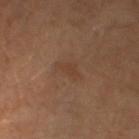Part of a total-body skin-imaging series; this lesion was reviewed on a skin check and was not flagged for biopsy.
A male patient, aged around 30.
Automated image analysis of the tile measured a mean CIELAB color near L≈38 a*≈18 b*≈28, a lesion–skin lightness drop of about 5, and a normalized border contrast of about 5. It also reported a border-irregularity index near 4.5/10, a within-lesion color-variation index near 1/10, and radial color variation of about 0.5.
Located on the left lower leg.
Measured at roughly 3 mm in maximum diameter.
This is a cross-polarized tile.
A 15 mm close-up tile from a total-body photography series done for melanoma screening.